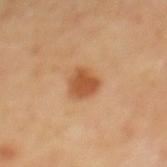Clinical impression: Part of a total-body skin-imaging series; this lesion was reviewed on a skin check and was not flagged for biopsy. Context: A 15 mm crop from a total-body photograph taken for skin-cancer surveillance. A male subject aged around 65. From the back.